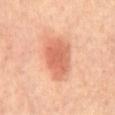Assessment:
Captured during whole-body skin photography for melanoma surveillance; the lesion was not biopsied.
Background:
This image is a 15 mm lesion crop taken from a total-body photograph. About 6 mm across. On the mid back.